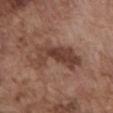A roughly 15 mm field-of-view crop from a total-body skin photograph. A male patient, aged 73 to 77. The recorded lesion diameter is about 7 mm. From the abdomen.